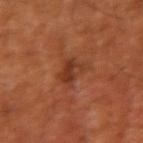Q: Was a biopsy performed?
A: total-body-photography surveillance lesion; no biopsy
Q: What lighting was used for the tile?
A: cross-polarized
Q: Lesion size?
A: about 3.5 mm
Q: What kind of image is this?
A: total-body-photography crop, ~15 mm field of view
Q: Patient demographics?
A: male, aged 58 to 62
Q: What is the anatomic site?
A: the right upper arm